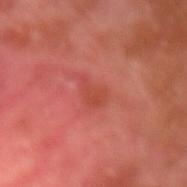Q: Was this lesion biopsied?
A: imaged on a skin check; not biopsied
Q: Patient demographics?
A: male, roughly 30 years of age
Q: What is the lesion's diameter?
A: ~3 mm (longest diameter)
Q: What is the anatomic site?
A: the left upper arm
Q: Automated lesion metrics?
A: border irregularity of about 4 on a 0–10 scale; a lesion-detection confidence of about 100/100
Q: How was this image acquired?
A: ~15 mm crop, total-body skin-cancer survey